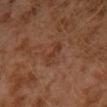The lesion was tiled from a total-body skin photograph and was not biopsied. The lesion is on the left leg. The lesion-visualizer software estimated a footprint of about 3.5 mm², an eccentricity of roughly 0.9, and a symmetry-axis asymmetry near 0.4. The software also gave a lesion-detection confidence of about 100/100. Longest diameter approximately 3 mm. A roughly 15 mm field-of-view crop from a total-body skin photograph. This is a cross-polarized tile. A male patient, approximately 30 years of age.